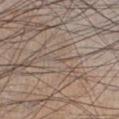Acquisition and patient details: Longest diameter approximately 1.5 mm. A male patient, in their mid-30s. The lesion-visualizer software estimated a lesion color around L≈52 a*≈11 b*≈22 in CIELAB, a lesion–skin lightness drop of about 5, and a normalized lesion–skin contrast near 3.5. And it measured a detector confidence of about 0 out of 100 that the crop contains a lesion. The tile uses white-light illumination. Cropped from a total-body skin-imaging series; the visible field is about 15 mm. Located on the right lower leg.